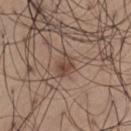Assessment: Recorded during total-body skin imaging; not selected for excision or biopsy. Clinical summary: A region of skin cropped from a whole-body photographic capture, roughly 15 mm wide. This is a white-light tile. The subject is a male about 65 years old. The lesion is on the left thigh. Automated tile analysis of the lesion measured a shape eccentricity near 0.65 and a symmetry-axis asymmetry near 0.35. The lesion's longest dimension is about 3 mm.